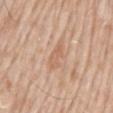follow-up=no biopsy performed (imaged during a skin exam)
image-analysis metrics=an area of roughly 5 mm², an eccentricity of roughly 0.85, and two-axis asymmetry of about 0.3
patient=male, in their mid-60s
lesion diameter=~3.5 mm (longest diameter)
image source=15 mm crop, total-body photography
body site=the mid back
lighting=white-light illumination